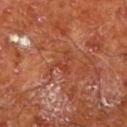subject: male, aged around 65; site: the left lower leg; illumination: cross-polarized; image source: total-body-photography crop, ~15 mm field of view.